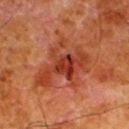Impression:
Recorded during total-body skin imaging; not selected for excision or biopsy.
Context:
Located on the left lower leg. A male subject, aged approximately 80. Captured under cross-polarized illumination. An algorithmic analysis of the crop reported a lesion–skin lightness drop of about 8. The software also gave border irregularity of about 7 on a 0–10 scale, a within-lesion color-variation index near 6.5/10, and peripheral color asymmetry of about 1.5. About 7 mm across. A lesion tile, about 15 mm wide, cut from a 3D total-body photograph.